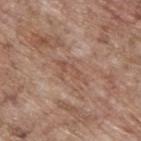Assessment:
Captured during whole-body skin photography for melanoma surveillance; the lesion was not biopsied.
Clinical summary:
The subject is a male aged around 70. About 4 mm across. The tile uses white-light illumination. A roughly 15 mm field-of-view crop from a total-body skin photograph. Automated tile analysis of the lesion measured a footprint of about 3.5 mm², an eccentricity of roughly 0.9, and a shape-asymmetry score of about 0.7 (0 = symmetric). The analysis additionally found a lesion–skin lightness drop of about 6 and a normalized lesion–skin contrast near 5. It also reported a border-irregularity index near 10/10 and internal color variation of about 0 on a 0–10 scale. The lesion is located on the upper back.